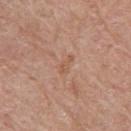Impression: Part of a total-body skin-imaging series; this lesion was reviewed on a skin check and was not flagged for biopsy. Image and clinical context: Imaged with white-light lighting. A region of skin cropped from a whole-body photographic capture, roughly 15 mm wide. The lesion-visualizer software estimated a lesion area of about 2.5 mm², a shape eccentricity near 0.9, and a symmetry-axis asymmetry near 0.3. And it measured a mean CIELAB color near L≈55 a*≈21 b*≈31, about 6 CIELAB-L* units darker than the surrounding skin, and a normalized lesion–skin contrast near 5. The patient is a male about 70 years old. On the right upper arm.